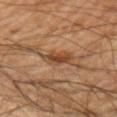{
  "biopsy_status": "not biopsied; imaged during a skin examination",
  "site": "left upper arm",
  "automated_metrics": {
    "area_mm2_approx": 5.5,
    "shape_asymmetry": 0.2,
    "cielab_L": 36,
    "cielab_a": 17,
    "cielab_b": 29,
    "vs_skin_contrast_norm": 7.5,
    "border_irregularity_0_10": 2.5,
    "color_variation_0_10": 4.0,
    "nevus_likeness_0_100": 75,
    "lesion_detection_confidence_0_100": 100
  },
  "image": {
    "source": "total-body photography crop",
    "field_of_view_mm": 15
  },
  "patient": {
    "sex": "male",
    "age_approx": 65
  },
  "lesion_size": {
    "long_diameter_mm_approx": 3.0
  }
}